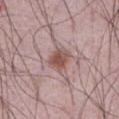Context:
Located on the abdomen. A male subject aged 63 to 67. About 3 mm across. A region of skin cropped from a whole-body photographic capture, roughly 15 mm wide. This is a white-light tile.